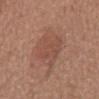follow-up: no biopsy performed (imaged during a skin exam) | image source: ~15 mm crop, total-body skin-cancer survey | lesion size: ≈5 mm | patient: male, aged approximately 65 | anatomic site: the mid back | tile lighting: white-light | image-analysis metrics: a lesion area of about 15 mm², a shape eccentricity near 0.55, and a shape-asymmetry score of about 0.3 (0 = symmetric); a lesion color around L≈49 a*≈22 b*≈27 in CIELAB and a normalized lesion–skin contrast near 4.5; border irregularity of about 3 on a 0–10 scale and a color-variation rating of about 2.5/10.